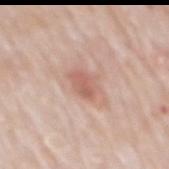Findings:
• notes — imaged on a skin check; not biopsied
• automated lesion analysis — a nevus-likeness score of about 85/100 and a detector confidence of about 100 out of 100 that the crop contains a lesion
• image source — ~15 mm crop, total-body skin-cancer survey
• size — ≈3 mm
• body site — the mid back
• patient — male, roughly 80 years of age
• lighting — white-light illumination